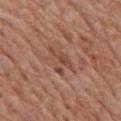Case summary:
* workup — catalogued during a skin exam; not biopsied
* anatomic site — the chest
* image — ~15 mm crop, total-body skin-cancer survey
* subject — male, aged around 65